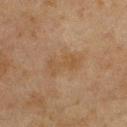Impression:
Imaged during a routine full-body skin examination; the lesion was not biopsied and no histopathology is available.
Image and clinical context:
The recorded lesion diameter is about 4.5 mm. A male subject, about 75 years old. From the chest. A region of skin cropped from a whole-body photographic capture, roughly 15 mm wide.